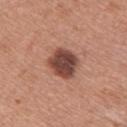Impression: Recorded during total-body skin imaging; not selected for excision or biopsy. Image and clinical context: A female subject roughly 35 years of age. The total-body-photography lesion software estimated a border-irregularity index near 2/10, internal color variation of about 4 on a 0–10 scale, and a peripheral color-asymmetry measure near 1. The software also gave an automated nevus-likeness rating near 80 out of 100 and a detector confidence of about 100 out of 100 that the crop contains a lesion. On the upper back. A 15 mm close-up extracted from a 3D total-body photography capture. The tile uses white-light illumination. The recorded lesion diameter is about 4 mm.